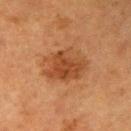Impression:
Part of a total-body skin-imaging series; this lesion was reviewed on a skin check and was not flagged for biopsy.
Context:
Captured under cross-polarized illumination. Automated image analysis of the tile measured a lesion color around L≈39 a*≈22 b*≈32 in CIELAB. The software also gave a border-irregularity rating of about 2.5/10 and internal color variation of about 4 on a 0–10 scale. A female subject, in their mid- to late 50s. This image is a 15 mm lesion crop taken from a total-body photograph. On the right upper arm. The recorded lesion diameter is about 5.5 mm.